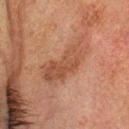Captured during whole-body skin photography for melanoma surveillance; the lesion was not biopsied.